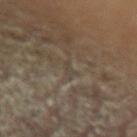workup: catalogued during a skin exam; not biopsied | location: the left forearm | subject: male, about 70 years old | image-analysis metrics: internal color variation of about 0 on a 0–10 scale and peripheral color asymmetry of about 0 | image source: 15 mm crop, total-body photography.